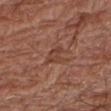Notes:
* biopsy status: no biopsy performed (imaged during a skin exam)
* patient: female, in their 80s
* tile lighting: white-light illumination
* site: the right forearm
* lesion size: about 3 mm
* imaging modality: total-body-photography crop, ~15 mm field of view